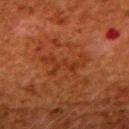This lesion was catalogued during total-body skin photography and was not selected for biopsy. Imaged with cross-polarized lighting. A female patient roughly 50 years of age. Automated tile analysis of the lesion measured a footprint of about 8 mm², an eccentricity of roughly 0.9, and a shape-asymmetry score of about 0.65 (0 = symmetric). The software also gave a mean CIELAB color near L≈30 a*≈25 b*≈32 and a normalized lesion–skin contrast near 5. And it measured border irregularity of about 10 on a 0–10 scale, a within-lesion color-variation index near 0.5/10, and a peripheral color-asymmetry measure near 0. A lesion tile, about 15 mm wide, cut from a 3D total-body photograph. The recorded lesion diameter is about 5.5 mm. From the upper back.